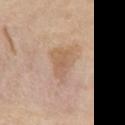  biopsy_status: not biopsied; imaged during a skin examination
  patient:
    sex: female
    age_approx: 25
  image:
    source: total-body photography crop
    field_of_view_mm: 15
  site: upper back